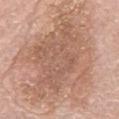<lesion>
  <biopsy_status>not biopsied; imaged during a skin examination</biopsy_status>
  <automated_metrics>
    <area_mm2_approx>95.0</area_mm2_approx>
    <eccentricity>0.7</eccentricity>
    <border_irregularity_0_10>6.5</border_irregularity_0_10>
    <color_variation_0_10>5.0</color_variation_0_10>
  </automated_metrics>
  <lesion_size>
    <long_diameter_mm_approx>14.5</long_diameter_mm_approx>
  </lesion_size>
  <image>
    <source>total-body photography crop</source>
    <field_of_view_mm>15</field_of_view_mm>
  </image>
  <patient>
    <sex>male</sex>
    <age_approx>80</age_approx>
  </patient>
  <site>mid back</site>
</lesion>